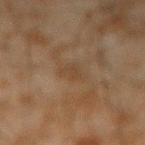Case summary:
• follow-up — imaged on a skin check; not biopsied
• subject — male, aged approximately 45
• location — the left forearm
• acquisition — 15 mm crop, total-body photography
• lesion diameter — ≈3 mm